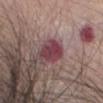Imaged during a routine full-body skin examination; the lesion was not biopsied and no histopathology is available. Measured at roughly 3.5 mm in maximum diameter. The patient is a female aged approximately 65. A 15 mm close-up tile from a total-body photography series done for melanoma screening. The lesion is on the head or neck. An algorithmic analysis of the crop reported a mean CIELAB color near L≈40 a*≈26 b*≈15, a lesion–skin lightness drop of about 13, and a lesion-to-skin contrast of about 10.5 (normalized; higher = more distinct). The analysis additionally found a border-irregularity rating of about 2/10 and radial color variation of about 2.